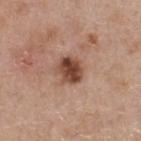Part of a total-body skin-imaging series; this lesion was reviewed on a skin check and was not flagged for biopsy. A male patient approximately 55 years of age. This is a white-light tile. A region of skin cropped from a whole-body photographic capture, roughly 15 mm wide. Approximately 3 mm at its widest.